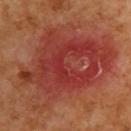follow-up: no biopsy performed (imaged during a skin exam)
anatomic site: the back
subject: male, aged approximately 60
image: ~15 mm tile from a whole-body skin photo